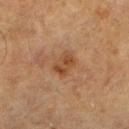| feature | finding |
|---|---|
| notes | total-body-photography surveillance lesion; no biopsy |
| image | ~15 mm crop, total-body skin-cancer survey |
| site | the right lower leg |
| diameter | ~2.5 mm (longest diameter) |
| patient | male, in their mid-60s |